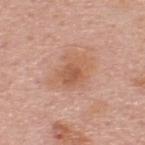Part of a total-body skin-imaging series; this lesion was reviewed on a skin check and was not flagged for biopsy.
Automated image analysis of the tile measured about 8 CIELAB-L* units darker than the surrounding skin.
Captured under white-light illumination.
The lesion is on the upper back.
Approximately 4.5 mm at its widest.
The subject is a male aged approximately 65.
A close-up tile cropped from a whole-body skin photograph, about 15 mm across.